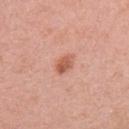{
  "biopsy_status": "not biopsied; imaged during a skin examination",
  "image": {
    "source": "total-body photography crop",
    "field_of_view_mm": 15
  },
  "site": "right upper arm",
  "patient": {
    "sex": "female",
    "age_approx": 35
  },
  "lighting": "white-light",
  "lesion_size": {
    "long_diameter_mm_approx": 2.5
  }
}